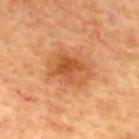Clinical impression: Recorded during total-body skin imaging; not selected for excision or biopsy. Acquisition and patient details: Longest diameter approximately 5 mm. From the upper back. An algorithmic analysis of the crop reported an area of roughly 15 mm², an eccentricity of roughly 0.7, and two-axis asymmetry of about 0.25. It also reported a classifier nevus-likeness of about 80/100 and lesion-presence confidence of about 100/100. A male patient, in their 60s. A lesion tile, about 15 mm wide, cut from a 3D total-body photograph.